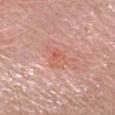Clinical impression:
This lesion was catalogued during total-body skin photography and was not selected for biopsy.
Image and clinical context:
The patient is a female about 40 years old. This is a white-light tile. Cropped from a total-body skin-imaging series; the visible field is about 15 mm. The lesion-visualizer software estimated a classifier nevus-likeness of about 0/100 and lesion-presence confidence of about 100/100. The lesion is located on the head or neck. Longest diameter approximately 4 mm.